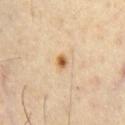{"biopsy_status": "not biopsied; imaged during a skin examination", "image": {"source": "total-body photography crop", "field_of_view_mm": 15}, "patient": {"sex": "male", "age_approx": 65}, "site": "chest"}